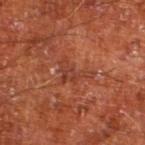Context: The subject is a male about 65 years old. Located on the left lower leg. The tile uses cross-polarized illumination. Longest diameter approximately 4 mm. A 15 mm close-up extracted from a 3D total-body photography capture.